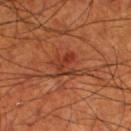Impression: Part of a total-body skin-imaging series; this lesion was reviewed on a skin check and was not flagged for biopsy. Clinical summary: On the leg. Automated image analysis of the tile measured a within-lesion color-variation index near 4.5/10. The lesion's longest dimension is about 3.5 mm. A male patient, about 70 years old. A 15 mm close-up tile from a total-body photography series done for melanoma screening. This is a cross-polarized tile.